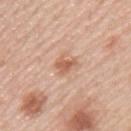| feature | finding |
|---|---|
| workup | total-body-photography surveillance lesion; no biopsy |